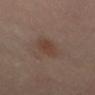From the front of the torso. This is a cross-polarized tile. Automated image analysis of the tile measured a classifier nevus-likeness of about 35/100 and lesion-presence confidence of about 100/100. A female subject, aged around 50. This image is a 15 mm lesion crop taken from a total-body photograph.